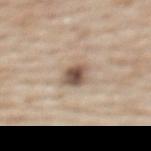{
  "biopsy_status": "not biopsied; imaged during a skin examination",
  "site": "upper back",
  "lighting": "white-light",
  "lesion_size": {
    "long_diameter_mm_approx": 3.0
  },
  "image": {
    "source": "total-body photography crop",
    "field_of_view_mm": 15
  },
  "patient": {
    "sex": "female",
    "age_approx": 50
  },
  "automated_metrics": {
    "cielab_L": 53,
    "cielab_a": 14,
    "cielab_b": 26,
    "vs_skin_darker_L": 15.0,
    "nevus_likeness_0_100": 90,
    "lesion_detection_confidence_0_100": 100
  }
}